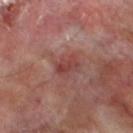Recorded during total-body skin imaging; not selected for excision or biopsy. The lesion is located on the left lower leg. The subject is a male in their 70s. A 15 mm crop from a total-body photograph taken for skin-cancer surveillance.